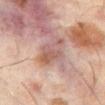{
  "biopsy_status": "not biopsied; imaged during a skin examination",
  "patient": {
    "sex": "male",
    "age_approx": 60
  },
  "image": {
    "source": "total-body photography crop",
    "field_of_view_mm": 15
  },
  "site": "abdomen"
}